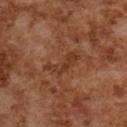<case>
<biopsy_status>not biopsied; imaged during a skin examination</biopsy_status>
<lighting>cross-polarized</lighting>
<automated_metrics>
  <cielab_L>32</cielab_L>
  <cielab_a>21</cielab_a>
  <cielab_b>28</cielab_b>
  <vs_skin_darker_L>6.0</vs_skin_darker_L>
  <vs_skin_contrast_norm>6.0</vs_skin_contrast_norm>
  <nevus_likeness_0_100>0</nevus_likeness_0_100>
  <lesion_detection_confidence_0_100>85</lesion_detection_confidence_0_100>
</automated_metrics>
<lesion_size>
  <long_diameter_mm_approx>5.0</long_diameter_mm_approx>
</lesion_size>
<image>
  <source>total-body photography crop</source>
  <field_of_view_mm>15</field_of_view_mm>
</image>
<site>upper back</site>
<patient>
  <sex>female</sex>
  <age_approx>60</age_approx>
</patient>
</case>